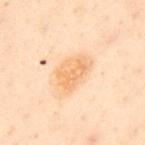Findings:
• image-analysis metrics: an area of roughly 10 mm², an eccentricity of roughly 0.8, and a symmetry-axis asymmetry near 0.3; about 7 CIELAB-L* units darker than the surrounding skin and a normalized border contrast of about 6.5; a border-irregularity index near 3/10, internal color variation of about 2.5 on a 0–10 scale, and a peripheral color-asymmetry measure near 1; an automated nevus-likeness rating near 75 out of 100 and a detector confidence of about 100 out of 100 that the crop contains a lesion
• lighting: cross-polarized
• diameter: ≈4.5 mm
• subject: male, aged 48 to 52
• image source: ~15 mm tile from a whole-body skin photo
• site: the upper back